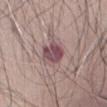Assessment:
This lesion was catalogued during total-body skin photography and was not selected for biopsy.
Image and clinical context:
The lesion is on the front of the torso. A 15 mm close-up extracted from a 3D total-body photography capture. A male subject, aged around 65.